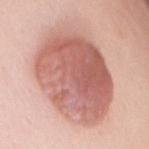| field | value |
|---|---|
| biopsy status | total-body-photography surveillance lesion; no biopsy |